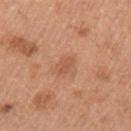A female subject, aged approximately 40. On the arm. A close-up tile cropped from a whole-body skin photograph, about 15 mm across.